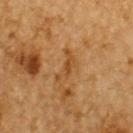biopsy status: total-body-photography surveillance lesion; no biopsy
acquisition: 15 mm crop, total-body photography
diameter: ~4 mm (longest diameter)
site: the upper back
subject: male, in their mid-80s or older
image-analysis metrics: an area of roughly 4 mm², an outline eccentricity of about 0.9 (0 = round, 1 = elongated), and a symmetry-axis asymmetry near 0.75; a border-irregularity rating of about 7.5/10 and a peripheral color-asymmetry measure near 0; a classifier nevus-likeness of about 0/100 and a lesion-detection confidence of about 65/100